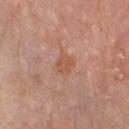Impression:
Imaged during a routine full-body skin examination; the lesion was not biopsied and no histopathology is available.
Image and clinical context:
The tile uses white-light illumination. A 15 mm crop from a total-body photograph taken for skin-cancer surveillance. The subject is a male about 75 years old. The lesion's longest dimension is about 2.5 mm. The lesion is located on the chest. Automated tile analysis of the lesion measured border irregularity of about 2.5 on a 0–10 scale, a within-lesion color-variation index near 2.5/10, and radial color variation of about 1. And it measured an automated nevus-likeness rating near 5 out of 100 and lesion-presence confidence of about 100/100.